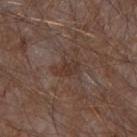biopsy status: total-body-photography surveillance lesion; no biopsy
patient: male, in their mid- to late 70s
lesion diameter: ~2.5 mm (longest diameter)
lighting: white-light
body site: the right forearm
imaging modality: 15 mm crop, total-body photography